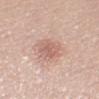Impression: Captured during whole-body skin photography for melanoma surveillance; the lesion was not biopsied. Background: A 15 mm crop from a total-body photograph taken for skin-cancer surveillance. A female patient, roughly 60 years of age. Located on the leg.